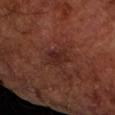Impression: Captured during whole-body skin photography for melanoma surveillance; the lesion was not biopsied. Context: From the arm. A male patient approximately 65 years of age. A 15 mm close-up tile from a total-body photography series done for melanoma screening.